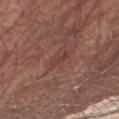This lesion was catalogued during total-body skin photography and was not selected for biopsy.
On the right thigh.
The lesion-visualizer software estimated a footprint of about 3 mm², an eccentricity of roughly 0.95, and two-axis asymmetry of about 0.4. It also reported a nevus-likeness score of about 0/100 and a lesion-detection confidence of about 60/100.
The lesion's longest dimension is about 3 mm.
A region of skin cropped from a whole-body photographic capture, roughly 15 mm wide.
Imaged with white-light lighting.
The subject is a male aged around 55.